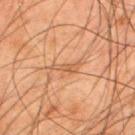A lesion tile, about 15 mm wide, cut from a 3D total-body photograph.
Imaged with cross-polarized lighting.
Measured at roughly 3.5 mm in maximum diameter.
The lesion is on the upper back.
A male patient about 45 years old.